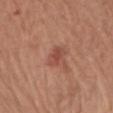| key | value |
|---|---|
| notes | imaged on a skin check; not biopsied |
| diameter | ≈2.5 mm |
| body site | the chest |
| subject | female, aged approximately 75 |
| automated lesion analysis | a footprint of about 4.5 mm², an outline eccentricity of about 0.75 (0 = round, 1 = elongated), and a symmetry-axis asymmetry near 0.25; a border-irregularity rating of about 2/10, internal color variation of about 2 on a 0–10 scale, and peripheral color asymmetry of about 0.5; a nevus-likeness score of about 20/100 and a lesion-detection confidence of about 100/100 |
| acquisition | total-body-photography crop, ~15 mm field of view |
| tile lighting | white-light |